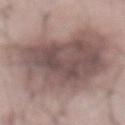notes: no biopsy performed (imaged during a skin exam) | acquisition: total-body-photography crop, ~15 mm field of view | TBP lesion metrics: a footprint of about 75 mm² and a shape eccentricity near 0.65; a nevus-likeness score of about 30/100 and a detector confidence of about 100 out of 100 that the crop contains a lesion | patient: male, aged 53 to 57 | size: about 11.5 mm | tile lighting: white-light illumination | anatomic site: the abdomen.